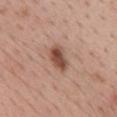Case summary:
• notes: catalogued during a skin exam; not biopsied
• lesion diameter: ≈4 mm
• illumination: white-light
• subject: female, aged around 45
• imaging modality: total-body-photography crop, ~15 mm field of view
• site: the front of the torso
• automated lesion analysis: an area of roughly 6.5 mm², an outline eccentricity of about 0.8 (0 = round, 1 = elongated), and a shape-asymmetry score of about 0.15 (0 = symmetric); an average lesion color of about L≈50 a*≈21 b*≈28 (CIELAB), a lesion–skin lightness drop of about 13, and a lesion-to-skin contrast of about 9.5 (normalized; higher = more distinct); a classifier nevus-likeness of about 90/100